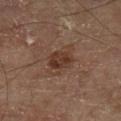Imaged during a routine full-body skin examination; the lesion was not biopsied and no histopathology is available. Located on the left lower leg. A 15 mm close-up extracted from a 3D total-body photography capture. The recorded lesion diameter is about 4.5 mm. A male subject, about 70 years old.